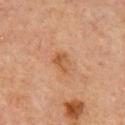Case summary:
• lighting — cross-polarized illumination
• TBP lesion metrics — an automated nevus-likeness rating near 10 out of 100 and lesion-presence confidence of about 100/100
• anatomic site — the back
• subject — male, in their mid-60s
• image source — ~15 mm crop, total-body skin-cancer survey